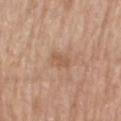{"biopsy_status": "not biopsied; imaged during a skin examination", "lighting": "white-light", "image": {"source": "total-body photography crop", "field_of_view_mm": 15}, "lesion_size": {"long_diameter_mm_approx": 2.5}, "site": "left thigh", "patient": {"sex": "male", "age_approx": 70}, "automated_metrics": {"area_mm2_approx": 3.5, "eccentricity": 0.8, "shape_asymmetry": 0.3, "nevus_likeness_0_100": 0, "lesion_detection_confidence_0_100": 100}}